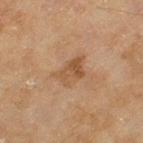Longest diameter approximately 4 mm.
A female patient aged around 60.
Located on the leg.
Cropped from a whole-body photographic skin survey; the tile spans about 15 mm.